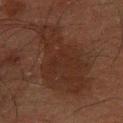This lesion was catalogued during total-body skin photography and was not selected for biopsy. The lesion is on the left forearm. The subject is a male aged 58–62. A 15 mm close-up extracted from a 3D total-body photography capture.